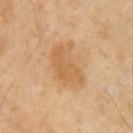workup=total-body-photography surveillance lesion; no biopsy
body site=the chest
patient=male, aged 63–67
image=~15 mm crop, total-body skin-cancer survey